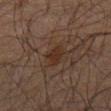Recorded during total-body skin imaging; not selected for excision or biopsy.